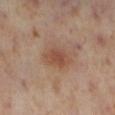Q: Was this lesion biopsied?
A: imaged on a skin check; not biopsied
Q: What kind of image is this?
A: total-body-photography crop, ~15 mm field of view
Q: What is the lesion's diameter?
A: ≈4 mm
Q: Where on the body is the lesion?
A: the left lower leg
Q: Who is the patient?
A: female, in their mid-50s